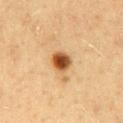The lesion was tiled from a total-body skin photograph and was not biopsied.
Captured under cross-polarized illumination.
An algorithmic analysis of the crop reported an average lesion color of about L≈44 a*≈21 b*≈36 (CIELAB), roughly 17 lightness units darker than nearby skin, and a normalized border contrast of about 12. The analysis additionally found a classifier nevus-likeness of about 100/100.
From the mid back.
The subject is a female aged 48 to 52.
A close-up tile cropped from a whole-body skin photograph, about 15 mm across.